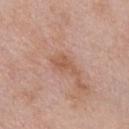{"biopsy_status": "not biopsied; imaged during a skin examination", "automated_metrics": {"area_mm2_approx": 3.5, "eccentricity": 0.85, "shape_asymmetry": 0.45, "vs_skin_darker_L": 8.0, "border_irregularity_0_10": 5.0, "color_variation_0_10": 1.5, "peripheral_color_asymmetry": 0.5, "nevus_likeness_0_100": 0, "lesion_detection_confidence_0_100": 100}, "image": {"source": "total-body photography crop", "field_of_view_mm": 15}, "lesion_size": {"long_diameter_mm_approx": 3.0}, "lighting": "white-light", "site": "chest", "patient": {"sex": "male", "age_approx": 55}}